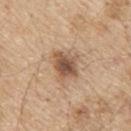follow-up: catalogued during a skin exam; not biopsied | patient: male, roughly 70 years of age | image: 15 mm crop, total-body photography | lesion size: about 4 mm | anatomic site: the upper back | image-analysis metrics: a lesion color around L≈54 a*≈18 b*≈31 in CIELAB, roughly 13 lightness units darker than nearby skin, and a normalized border contrast of about 8.5; internal color variation of about 5 on a 0–10 scale and a peripheral color-asymmetry measure near 1.5.